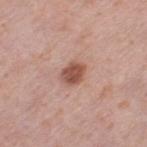No biopsy was performed on this lesion — it was imaged during a full skin examination and was not determined to be concerning.
This is a white-light tile.
A close-up tile cropped from a whole-body skin photograph, about 15 mm across.
A female subject, approximately 40 years of age.
From the leg.
Automated tile analysis of the lesion measured roughly 14 lightness units darker than nearby skin and a lesion-to-skin contrast of about 9.5 (normalized; higher = more distinct).